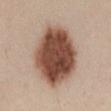The lesion was tiled from a total-body skin photograph and was not biopsied.
Imaged with white-light lighting.
The lesion is on the chest.
A female subject aged around 25.
A 15 mm crop from a total-body photograph taken for skin-cancer surveillance.
The lesion's longest dimension is about 8.5 mm.
An algorithmic analysis of the crop reported a within-lesion color-variation index near 7.5/10 and radial color variation of about 2.5. The software also gave a classifier nevus-likeness of about 100/100 and a detector confidence of about 100 out of 100 that the crop contains a lesion.